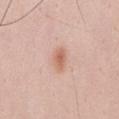No biopsy was performed on this lesion — it was imaged during a full skin examination and was not determined to be concerning. The lesion's longest dimension is about 3 mm. The tile uses white-light illumination. The lesion is on the chest. A male patient, aged around 35. A 15 mm close-up tile from a total-body photography series done for melanoma screening.